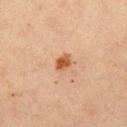biopsy status: imaged on a skin check; not biopsied
lighting: cross-polarized
lesion size: ≈2 mm
image source: ~15 mm tile from a whole-body skin photo
patient: male, roughly 60 years of age
body site: the left upper arm
automated metrics: a lesion color around L≈46 a*≈21 b*≈33 in CIELAB, roughly 11 lightness units darker than nearby skin, and a lesion-to-skin contrast of about 9.5 (normalized; higher = more distinct); a border-irregularity index near 2/10 and peripheral color asymmetry of about 0.5